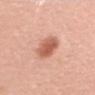Imaged during a routine full-body skin examination; the lesion was not biopsied and no histopathology is available.
The lesion is on the right upper arm.
Longest diameter approximately 4 mm.
The tile uses white-light illumination.
A lesion tile, about 15 mm wide, cut from a 3D total-body photograph.
The total-body-photography lesion software estimated a mean CIELAB color near L≈60 a*≈26 b*≈32, roughly 14 lightness units darker than nearby skin, and a lesion-to-skin contrast of about 8.5 (normalized; higher = more distinct). And it measured a border-irregularity rating of about 2.5/10, a color-variation rating of about 3.5/10, and a peripheral color-asymmetry measure near 1. It also reported a classifier nevus-likeness of about 95/100 and lesion-presence confidence of about 100/100.
A female patient aged 48 to 52.